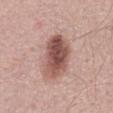  biopsy_status: not biopsied; imaged during a skin examination
  site: abdomen
  lesion_size:
    long_diameter_mm_approx: 6.0
  lighting: white-light
  automated_metrics:
    border_irregularity_0_10: 2.0
    nevus_likeness_0_100: 95
  patient:
    sex: male
    age_approx: 60
  image:
    source: total-body photography crop
    field_of_view_mm: 15